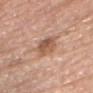  biopsy_status: not biopsied; imaged during a skin examination
  lighting: white-light
  site: head or neck
  image:
    source: total-body photography crop
    field_of_view_mm: 15
  lesion_size:
    long_diameter_mm_approx: 4.5
  patient:
    sex: male
    age_approx: 80